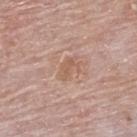The lesion was tiled from a total-body skin photograph and was not biopsied. The patient is a male roughly 85 years of age or older. This is a white-light tile. A 15 mm close-up extracted from a 3D total-body photography capture. The lesion is located on the upper back.